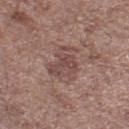Q: Was this lesion biopsied?
A: imaged on a skin check; not biopsied
Q: Where on the body is the lesion?
A: the leg
Q: Who is the patient?
A: male, aged approximately 70
Q: What lighting was used for the tile?
A: white-light
Q: What is the imaging modality?
A: ~15 mm tile from a whole-body skin photo
Q: How large is the lesion?
A: about 4 mm